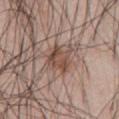<tbp_lesion>
<patient>
  <sex>male</sex>
  <age_approx>70</age_approx>
</patient>
<site>abdomen</site>
<lighting>white-light</lighting>
<lesion_size>
  <long_diameter_mm_approx>3.5</long_diameter_mm_approx>
</lesion_size>
<image>
  <source>total-body photography crop</source>
  <field_of_view_mm>15</field_of_view_mm>
</image>
</tbp_lesion>